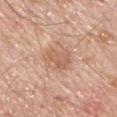<lesion>
<biopsy_status>not biopsied; imaged during a skin examination</biopsy_status>
<site>front of the torso</site>
<lighting>white-light</lighting>
<patient>
  <sex>male</sex>
  <age_approx>60</age_approx>
</patient>
<lesion_size>
  <long_diameter_mm_approx>4.0</long_diameter_mm_approx>
</lesion_size>
<image>
  <source>total-body photography crop</source>
  <field_of_view_mm>15</field_of_view_mm>
</image>
</lesion>